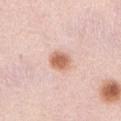Clinical impression: This lesion was catalogued during total-body skin photography and was not selected for biopsy. Context: This is a white-light tile. A female patient aged approximately 40. An algorithmic analysis of the crop reported a mean CIELAB color near L≈65 a*≈23 b*≈31, a lesion–skin lightness drop of about 14, and a lesion-to-skin contrast of about 9.5 (normalized; higher = more distinct). Cropped from a whole-body photographic skin survey; the tile spans about 15 mm. The lesion is located on the abdomen. Longest diameter approximately 3 mm.